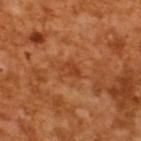Captured during whole-body skin photography for melanoma surveillance; the lesion was not biopsied. Captured under cross-polarized illumination. A male patient aged approximately 65. Cropped from a total-body skin-imaging series; the visible field is about 15 mm. Measured at roughly 2.5 mm in maximum diameter.